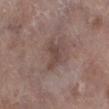Impression: No biopsy was performed on this lesion — it was imaged during a full skin examination and was not determined to be concerning. Background: A female patient, in their mid- to late 80s or older. Located on the left lower leg. Captured under white-light illumination. The lesion's longest dimension is about 3.5 mm. This image is a 15 mm lesion crop taken from a total-body photograph.